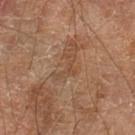  biopsy_status: not biopsied; imaged during a skin examination
  image:
    source: total-body photography crop
    field_of_view_mm: 15
  lighting: cross-polarized
  site: left lower leg
  lesion_size:
    long_diameter_mm_approx: 3.5
  patient:
    sex: male
    age_approx: 70